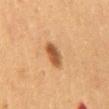Captured under cross-polarized illumination. Measured at roughly 3.5 mm in maximum diameter. A female patient aged 48–52. A roughly 15 mm field-of-view crop from a total-body skin photograph. On the front of the torso.